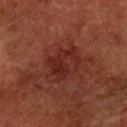Q: Was a biopsy performed?
A: total-body-photography surveillance lesion; no biopsy
Q: What are the patient's age and sex?
A: in their mid-60s
Q: Lesion size?
A: about 4.5 mm
Q: What kind of image is this?
A: 15 mm crop, total-body photography
Q: What is the anatomic site?
A: the left forearm